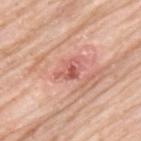Assessment:
Imaged during a routine full-body skin examination; the lesion was not biopsied and no histopathology is available.
Clinical summary:
The tile uses white-light illumination. A roughly 15 mm field-of-view crop from a total-body skin photograph. Automated tile analysis of the lesion measured a footprint of about 3.5 mm² and a shape-asymmetry score of about 0.45 (0 = symmetric). It also reported a nevus-likeness score of about 0/100 and a detector confidence of about 100 out of 100 that the crop contains a lesion. Approximately 3 mm at its widest. A male subject in their 80s. From the upper back.